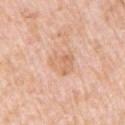Notes:
• notes · no biopsy performed (imaged during a skin exam)
• location · the arm
• image source · ~15 mm crop, total-body skin-cancer survey
• diameter · about 3.5 mm
• tile lighting · white-light illumination
• patient · male, in their 50s